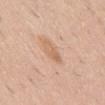The lesion was photographed on a routine skin check and not biopsied; there is no pathology result. Imaged with white-light lighting. The recorded lesion diameter is about 3.5 mm. A male patient, aged around 40. The lesion is located on the front of the torso. The total-body-photography lesion software estimated an area of roughly 5 mm², an outline eccentricity of about 0.9 (0 = round, 1 = elongated), and a shape-asymmetry score of about 0.3 (0 = symmetric). The analysis additionally found a mean CIELAB color near L≈64 a*≈20 b*≈33, about 8 CIELAB-L* units darker than the surrounding skin, and a normalized border contrast of about 5.5. A 15 mm close-up tile from a total-body photography series done for melanoma screening.